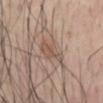• workup · catalogued during a skin exam; not biopsied
• anatomic site · the abdomen
• acquisition · 15 mm crop, total-body photography
• lesion diameter · about 3.5 mm
• tile lighting · white-light
• automated lesion analysis · an outline eccentricity of about 0.85 (0 = round, 1 = elongated) and a symmetry-axis asymmetry near 0.25
• subject · male, roughly 55 years of age